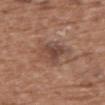The recorded lesion diameter is about 3.5 mm.
The lesion-visualizer software estimated a shape eccentricity near 0.65. The software also gave an automated nevus-likeness rating near 5 out of 100 and a lesion-detection confidence of about 100/100.
Located on the upper back.
The patient is a female in their mid-70s.
Cropped from a whole-body photographic skin survey; the tile spans about 15 mm.